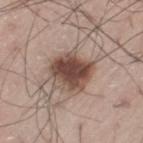Impression:
The lesion was photographed on a routine skin check and not biopsied; there is no pathology result.
Acquisition and patient details:
The subject is a male in their 70s. The tile uses white-light illumination. The lesion is on the lower back. A 15 mm close-up extracted from a 3D total-body photography capture.